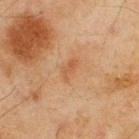Clinical impression:
The lesion was photographed on a routine skin check and not biopsied; there is no pathology result.
Background:
Approximately 2.5 mm at its widest. A 15 mm close-up extracted from a 3D total-body photography capture. Automated tile analysis of the lesion measured a mean CIELAB color near L≈45 a*≈20 b*≈31, roughly 6 lightness units darker than nearby skin, and a lesion-to-skin contrast of about 5 (normalized; higher = more distinct). It also reported border irregularity of about 5 on a 0–10 scale and internal color variation of about 0 on a 0–10 scale. Located on the upper back. A male subject aged 43 to 47. The tile uses cross-polarized illumination.